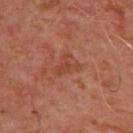Recorded during total-body skin imaging; not selected for excision or biopsy. Approximately 3 mm at its widest. Automated image analysis of the tile measured an outline eccentricity of about 0.7 (0 = round, 1 = elongated) and a shape-asymmetry score of about 0.6 (0 = symmetric). And it measured a border-irregularity index near 7.5/10, internal color variation of about 0.5 on a 0–10 scale, and peripheral color asymmetry of about 0. A 15 mm close-up tile from a total-body photography series done for melanoma screening. Captured under cross-polarized illumination. The lesion is on the upper back. A male subject about 50 years old.